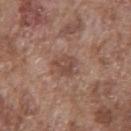Recorded during total-body skin imaging; not selected for excision or biopsy.
Approximately 3 mm at its widest.
Located on the chest.
This image is a 15 mm lesion crop taken from a total-body photograph.
The patient is a male in their mid-70s.